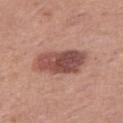{"biopsy_status": "not biopsied; imaged during a skin examination", "patient": {"sex": "female", "age_approx": 40}, "site": "leg", "lesion_size": {"long_diameter_mm_approx": 6.5}, "lighting": "white-light", "image": {"source": "total-body photography crop", "field_of_view_mm": 15}}